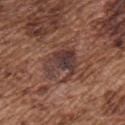notes: total-body-photography surveillance lesion; no biopsy
subject: male, in their mid-70s
size: ~5 mm (longest diameter)
tile lighting: white-light
anatomic site: the chest
image: 15 mm crop, total-body photography